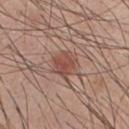Part of a total-body skin-imaging series; this lesion was reviewed on a skin check and was not flagged for biopsy.
A 15 mm close-up tile from a total-body photography series done for melanoma screening.
The lesion is located on the chest.
About 3.5 mm across.
A male subject aged around 25.
The tile uses white-light illumination.